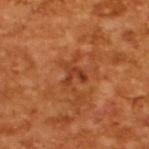Q: Was this lesion biopsied?
A: catalogued during a skin exam; not biopsied
Q: What kind of image is this?
A: total-body-photography crop, ~15 mm field of view
Q: Automated lesion metrics?
A: a lesion area of about 3.5 mm², a shape eccentricity near 0.55, and two-axis asymmetry of about 0.7; a border-irregularity index near 7.5/10 and a peripheral color-asymmetry measure near 0; a lesion-detection confidence of about 100/100
Q: How large is the lesion?
A: ~2.5 mm (longest diameter)
Q: What are the patient's age and sex?
A: male, aged 63 to 67